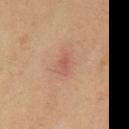The lesion was tiled from a total-body skin photograph and was not biopsied.
An algorithmic analysis of the crop reported a mean CIELAB color near L≈52 a*≈23 b*≈28, about 7 CIELAB-L* units darker than the surrounding skin, and a normalized border contrast of about 5. It also reported a border-irregularity rating of about 2.5/10, internal color variation of about 1 on a 0–10 scale, and a peripheral color-asymmetry measure near 0.5. It also reported a nevus-likeness score of about 0/100 and a detector confidence of about 100 out of 100 that the crop contains a lesion.
A male patient, roughly 60 years of age.
About 2.5 mm across.
From the chest.
A roughly 15 mm field-of-view crop from a total-body skin photograph.